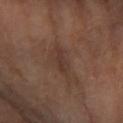Clinical impression: The lesion was tiled from a total-body skin photograph and was not biopsied. Image and clinical context: Located on the left forearm. Cropped from a whole-body photographic skin survey; the tile spans about 15 mm. This is a cross-polarized tile. About 3 mm across. A female subject approximately 70 years of age.